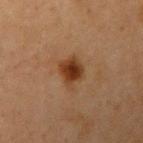Case summary:
– workup: catalogued during a skin exam; not biopsied
– location: the right upper arm
– tile lighting: cross-polarized illumination
– lesion diameter: ≈3.5 mm
– automated lesion analysis: a mean CIELAB color near L≈32 a*≈20 b*≈30, about 11 CIELAB-L* units darker than the surrounding skin, and a normalized border contrast of about 10.5; border irregularity of about 2.5 on a 0–10 scale and a color-variation rating of about 4.5/10; a classifier nevus-likeness of about 100/100 and lesion-presence confidence of about 100/100
– acquisition: ~15 mm crop, total-body skin-cancer survey
– patient: female, roughly 40 years of age